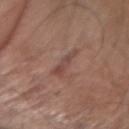<case>
<biopsy_status>not biopsied; imaged during a skin examination</biopsy_status>
<image>
  <source>total-body photography crop</source>
  <field_of_view_mm>15</field_of_view_mm>
</image>
<lesion_size>
  <long_diameter_mm_approx>3.5</long_diameter_mm_approx>
</lesion_size>
<site>arm</site>
<automated_metrics>
  <area_mm2_approx>4.0</area_mm2_approx>
  <eccentricity>0.95</eccentricity>
  <shape_asymmetry>0.35</shape_asymmetry>
</automated_metrics>
<lighting>white-light</lighting>
<patient>
  <sex>male</sex>
  <age_approx>70</age_approx>
</patient>
</case>